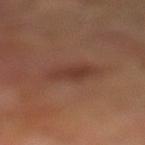{
  "biopsy_status": "not biopsied; imaged during a skin examination",
  "site": "left lower leg",
  "lighting": "cross-polarized",
  "lesion_size": {
    "long_diameter_mm_approx": 3.5
  },
  "image": {
    "source": "total-body photography crop",
    "field_of_view_mm": 15
  },
  "patient": {
    "sex": "male",
    "age_approx": 70
  }
}